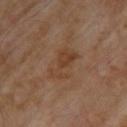| field | value |
|---|---|
| follow-up | catalogued during a skin exam; not biopsied |
| tile lighting | cross-polarized |
| site | the upper back |
| patient | male, roughly 70 years of age |
| imaging modality | ~15 mm tile from a whole-body skin photo |
| image-analysis metrics | a lesion area of about 8 mm² and a shape eccentricity near 0.8; a classifier nevus-likeness of about 0/100 and a lesion-detection confidence of about 100/100 |
| diameter | ≈4 mm |